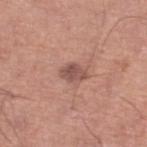– workup — imaged on a skin check; not biopsied
– acquisition — total-body-photography crop, ~15 mm field of view
– automated metrics — a border-irregularity index near 1.5/10, internal color variation of about 3 on a 0–10 scale, and peripheral color asymmetry of about 1; an automated nevus-likeness rating near 30 out of 100 and a lesion-detection confidence of about 100/100
– tile lighting — white-light
– lesion diameter — ≈3 mm
– anatomic site — the left lower leg
– patient — male, in their mid-60s